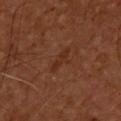Findings:
• notes · imaged on a skin check; not biopsied
• lighting · cross-polarized illumination
• image source · 15 mm crop, total-body photography
• location · the back
• lesion size · ~3.5 mm (longest diameter)
• TBP lesion metrics · a lesion–skin lightness drop of about 5 and a normalized lesion–skin contrast near 5.5; a nevus-likeness score of about 0/100 and a detector confidence of about 100 out of 100 that the crop contains a lesion
• subject · male, aged approximately 55